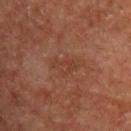Assessment:
Imaged during a routine full-body skin examination; the lesion was not biopsied and no histopathology is available.
Clinical summary:
The lesion's longest dimension is about 3.5 mm. The tile uses cross-polarized illumination. The patient is a male aged approximately 55. The lesion-visualizer software estimated a lesion area of about 3.5 mm² and a shape eccentricity near 0.85. Located on the upper back. A lesion tile, about 15 mm wide, cut from a 3D total-body photograph.